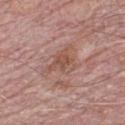{
  "biopsy_status": "not biopsied; imaged during a skin examination",
  "automated_metrics": {
    "area_mm2_approx": 8.0,
    "eccentricity": 0.65,
    "shape_asymmetry": 0.45
  },
  "image": {
    "source": "total-body photography crop",
    "field_of_view_mm": 15
  },
  "lighting": "white-light",
  "site": "chest",
  "lesion_size": {
    "long_diameter_mm_approx": 4.0
  },
  "patient": {
    "sex": "male",
    "age_approx": 70
  }
}